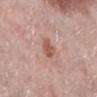Imaged during a routine full-body skin examination; the lesion was not biopsied and no histopathology is available. The lesion is located on the right lower leg. Cropped from a total-body skin-imaging series; the visible field is about 15 mm. A female patient aged approximately 65. This is a white-light tile.